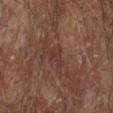{"biopsy_status": "not biopsied; imaged during a skin examination", "image": {"source": "total-body photography crop", "field_of_view_mm": 15}, "lighting": "cross-polarized", "lesion_size": {"long_diameter_mm_approx": 2.5}, "site": "right forearm", "patient": {"sex": "male", "age_approx": 65}}